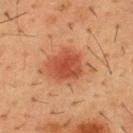Notes:
• biopsy status — total-body-photography surveillance lesion; no biopsy
• patient — male, about 55 years old
• body site — the front of the torso
• image source — total-body-photography crop, ~15 mm field of view